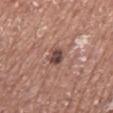notes: no biopsy performed (imaged during a skin exam) | TBP lesion metrics: a classifier nevus-likeness of about 10/100 and lesion-presence confidence of about 100/100 | subject: male, aged 73 to 77 | body site: the mid back | size: about 3 mm | acquisition: ~15 mm crop, total-body skin-cancer survey | lighting: white-light.